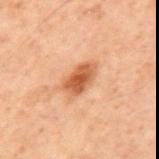No biopsy was performed on this lesion — it was imaged during a full skin examination and was not determined to be concerning. The lesion's longest dimension is about 4 mm. The subject is a male approximately 70 years of age. A close-up tile cropped from a whole-body skin photograph, about 15 mm across. The lesion is located on the mid back.